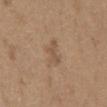biopsy status — total-body-photography surveillance lesion; no biopsy | automated lesion analysis — an area of roughly 4.5 mm², an eccentricity of roughly 0.85, and two-axis asymmetry of about 0.45; border irregularity of about 5 on a 0–10 scale; a nevus-likeness score of about 0/100 and a detector confidence of about 100 out of 100 that the crop contains a lesion | anatomic site — the chest | illumination — white-light | diameter — about 3 mm | image source — total-body-photography crop, ~15 mm field of view | subject — male, aged approximately 65.